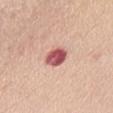Located on the abdomen.
A 15 mm close-up tile from a total-body photography series done for melanoma screening.
The patient is a female aged approximately 50.